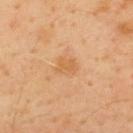Q: Was a biopsy performed?
A: no biopsy performed (imaged during a skin exam)
Q: How was this image acquired?
A: ~15 mm tile from a whole-body skin photo
Q: What lighting was used for the tile?
A: cross-polarized
Q: Lesion location?
A: the upper back
Q: Patient demographics?
A: male, roughly 40 years of age
Q: What did automated image analysis measure?
A: an outline eccentricity of about 0.6 (0 = round, 1 = elongated) and two-axis asymmetry of about 0.25; internal color variation of about 2.5 on a 0–10 scale and radial color variation of about 1; a nevus-likeness score of about 0/100 and a lesion-detection confidence of about 100/100
Q: What is the lesion's diameter?
A: ~3 mm (longest diameter)